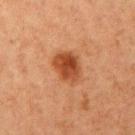follow-up: no biopsy performed (imaged during a skin exam) | size: ~4 mm (longest diameter) | location: the right upper arm | subject: female, roughly 40 years of age | acquisition: total-body-photography crop, ~15 mm field of view | lighting: cross-polarized.